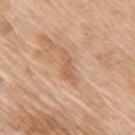follow-up: imaged on a skin check; not biopsied
size: ~4 mm (longest diameter)
site: the right upper arm
imaging modality: 15 mm crop, total-body photography
patient: female, roughly 75 years of age